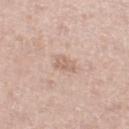This lesion was catalogued during total-body skin photography and was not selected for biopsy. The total-body-photography lesion software estimated a lesion area of about 4 mm², a shape eccentricity near 0.75, and a symmetry-axis asymmetry near 0.3. A male subject, in their mid-60s. Longest diameter approximately 2.5 mm. Cropped from a whole-body photographic skin survey; the tile spans about 15 mm. This is a white-light tile. Located on the left lower leg.